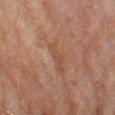Part of a total-body skin-imaging series; this lesion was reviewed on a skin check and was not flagged for biopsy. A female patient, in their 60s. A 15 mm close-up tile from a total-body photography series done for melanoma screening. This is a cross-polarized tile. Approximately 3.5 mm at its widest. The lesion is on the left lower leg.